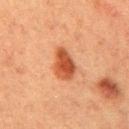Case summary:
– biopsy status — catalogued during a skin exam; not biopsied
– acquisition — ~15 mm tile from a whole-body skin photo
– automated lesion analysis — a mean CIELAB color near L≈43 a*≈26 b*≈34 and about 12 CIELAB-L* units darker than the surrounding skin
– body site — the right upper arm
– illumination — cross-polarized
– subject — female, aged around 55